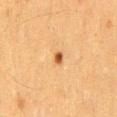The lesion was photographed on a routine skin check and not biopsied; there is no pathology result. The recorded lesion diameter is about 1.5 mm. A lesion tile, about 15 mm wide, cut from a 3D total-body photograph. From the back. A male subject aged approximately 35.